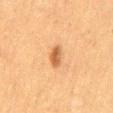Q: Was a biopsy performed?
A: total-body-photography surveillance lesion; no biopsy
Q: Who is the patient?
A: male, roughly 50 years of age
Q: What is the imaging modality?
A: 15 mm crop, total-body photography
Q: Lesion location?
A: the mid back